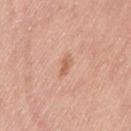Imaged during a routine full-body skin examination; the lesion was not biopsied and no histopathology is available. Captured under white-light illumination. The subject is a female in their mid- to late 60s. A region of skin cropped from a whole-body photographic capture, roughly 15 mm wide. The lesion is on the left thigh.